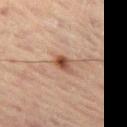biopsy status: catalogued during a skin exam; not biopsied
TBP lesion metrics: a footprint of about 3 mm² and a symmetry-axis asymmetry near 0.3; a lesion color around L≈45 a*≈21 b*≈29 in CIELAB and a lesion-to-skin contrast of about 9.5 (normalized; higher = more distinct); a border-irregularity index near 2.5/10 and peripheral color asymmetry of about 1.5; a nevus-likeness score of about 95/100 and a lesion-detection confidence of about 100/100
patient: male, in their 60s
lesion size: ~2.5 mm (longest diameter)
site: the left thigh
tile lighting: cross-polarized illumination
acquisition: 15 mm crop, total-body photography